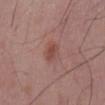| field | value |
|---|---|
| biopsy status | total-body-photography surveillance lesion; no biopsy |
| acquisition | total-body-photography crop, ~15 mm field of view |
| illumination | white-light |
| lesion size | ~3 mm (longest diameter) |
| body site | the mid back |
| automated metrics | a lesion color around L≈47 a*≈23 b*≈25 in CIELAB, about 8 CIELAB-L* units darker than the surrounding skin, and a normalized lesion–skin contrast near 6.5; a border-irregularity index near 2/10, a within-lesion color-variation index near 2.5/10, and radial color variation of about 1; a classifier nevus-likeness of about 75/100 and a lesion-detection confidence of about 100/100 |
| patient | male, aged 53 to 57 |